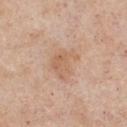notes = catalogued during a skin exam; not biopsied | image-analysis metrics = a footprint of about 9 mm², an eccentricity of roughly 0.7, and a symmetry-axis asymmetry near 0.4; a mean CIELAB color near L≈62 a*≈19 b*≈32; border irregularity of about 4.5 on a 0–10 scale | lesion size = ~4 mm (longest diameter) | imaging modality = total-body-photography crop, ~15 mm field of view | illumination = white-light illumination | body site = the chest | subject = male, in their mid- to late 50s.